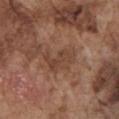Part of a total-body skin-imaging series; this lesion was reviewed on a skin check and was not flagged for biopsy.
Automated image analysis of the tile measured a lesion color around L≈43 a*≈19 b*≈27 in CIELAB and a normalized lesion–skin contrast near 5.5.
The tile uses white-light illumination.
A roughly 15 mm field-of-view crop from a total-body skin photograph.
Located on the chest.
Measured at roughly 4.5 mm in maximum diameter.
A male patient in their mid- to late 70s.